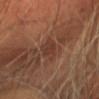Captured during whole-body skin photography for melanoma surveillance; the lesion was not biopsied.
Approximately 3.5 mm at its widest.
Located on the head or neck.
Captured under cross-polarized illumination.
A lesion tile, about 15 mm wide, cut from a 3D total-body photograph.
The patient is a male approximately 55 years of age.